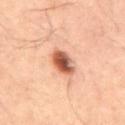TBP lesion metrics: a mean CIELAB color near L≈43 a*≈22 b*≈27 and a lesion-to-skin contrast of about 11.5 (normalized; higher = more distinct); a border-irregularity rating of about 2/10, internal color variation of about 5.5 on a 0–10 scale, and radial color variation of about 1.5; an automated nevus-likeness rating near 95 out of 100 and a lesion-detection confidence of about 100/100 | lighting: cross-polarized illumination | body site: the abdomen | lesion diameter: about 3.5 mm | image source: 15 mm crop, total-body photography | patient: male, in their 60s.